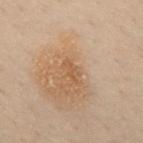Impression:
This lesion was catalogued during total-body skin photography and was not selected for biopsy.
Image and clinical context:
Automated tile analysis of the lesion measured an automated nevus-likeness rating near 0 out of 100. The patient is a male roughly 50 years of age. The lesion is located on the mid back. Captured under cross-polarized illumination. Longest diameter approximately 3 mm. A 15 mm close-up tile from a total-body photography series done for melanoma screening.